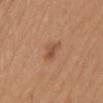  biopsy_status: not biopsied; imaged during a skin examination
  image:
    source: total-body photography crop
    field_of_view_mm: 15
  site: mid back
  patient:
    sex: female
    age_approx: 40
  lighting: white-light